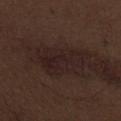Acquisition and patient details: A male subject, about 70 years old. Cropped from a total-body skin-imaging series; the visible field is about 15 mm. About 6 mm across. The lesion is on the abdomen. This is a white-light tile.